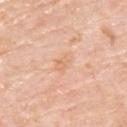The lesion was photographed on a routine skin check and not biopsied; there is no pathology result. Located on the upper back. Approximately 2.5 mm at its widest. This is a white-light tile. A 15 mm crop from a total-body photograph taken for skin-cancer surveillance. A male patient, aged 73–77.